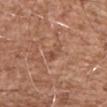No biopsy was performed on this lesion — it was imaged during a full skin examination and was not determined to be concerning.
Located on the chest.
Automated tile analysis of the lesion measured border irregularity of about 4 on a 0–10 scale and radial color variation of about 2.
A roughly 15 mm field-of-view crop from a total-body skin photograph.
A male subject approximately 55 years of age.
Measured at roughly 2.5 mm in maximum diameter.
Imaged with white-light lighting.